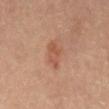The lesion was photographed on a routine skin check and not biopsied; there is no pathology result.
A roughly 15 mm field-of-view crop from a total-body skin photograph.
From the back.
The subject is a male in their mid-80s or older.